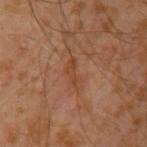Part of a total-body skin-imaging series; this lesion was reviewed on a skin check and was not flagged for biopsy. A 15 mm close-up extracted from a 3D total-body photography capture. The recorded lesion diameter is about 3.5 mm. Imaged with cross-polarized lighting. A male patient, in their 30s. From the arm.